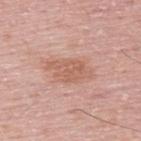Recorded during total-body skin imaging; not selected for excision or biopsy. Approximately 5.5 mm at its widest. Located on the upper back. A male subject about 50 years old. Cropped from a total-body skin-imaging series; the visible field is about 15 mm.